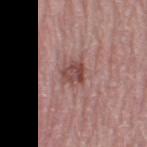Findings:
• size — about 2.5 mm
• patient — female, aged approximately 65
• imaging modality — total-body-photography crop, ~15 mm field of view
• tile lighting — white-light illumination
• location — the leg